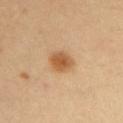illumination: cross-polarized illumination; patient: female, aged 33–37; image source: 15 mm crop, total-body photography; site: the front of the torso.